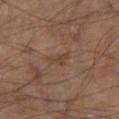This lesion was catalogued during total-body skin photography and was not selected for biopsy.
A roughly 15 mm field-of-view crop from a total-body skin photograph.
A male subject, aged around 70.
The lesion is located on the left lower leg.